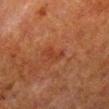Acquisition and patient details:
Located on the right lower leg. A male patient in their 80s. A 15 mm close-up tile from a total-body photography series done for melanoma screening. The recorded lesion diameter is about 3 mm. Imaged with cross-polarized lighting. Automated tile analysis of the lesion measured a lesion area of about 3 mm² and a shape eccentricity near 0.9. And it measured an average lesion color of about L≈31 a*≈23 b*≈29 (CIELAB) and roughly 5 lightness units darker than nearby skin.